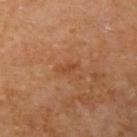Q: Is there a histopathology result?
A: catalogued during a skin exam; not biopsied
Q: Who is the patient?
A: male, approximately 55 years of age
Q: What lighting was used for the tile?
A: cross-polarized illumination
Q: How was this image acquired?
A: ~15 mm tile from a whole-body skin photo
Q: What is the lesion's diameter?
A: ~2.5 mm (longest diameter)
Q: Where on the body is the lesion?
A: the left upper arm